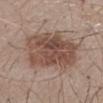Impression: Recorded during total-body skin imaging; not selected for excision or biopsy. Image and clinical context: This is a white-light tile. A male patient approximately 55 years of age. This image is a 15 mm lesion crop taken from a total-body photograph. Located on the mid back. About 7.5 mm across. The lesion-visualizer software estimated a lesion area of about 34 mm² and a symmetry-axis asymmetry near 0.25. It also reported border irregularity of about 3.5 on a 0–10 scale, internal color variation of about 5 on a 0–10 scale, and peripheral color asymmetry of about 2.